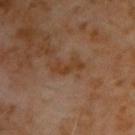This lesion was catalogued during total-body skin photography and was not selected for biopsy.
The lesion is on the right upper arm.
Cropped from a total-body skin-imaging series; the visible field is about 15 mm.
A male patient, aged around 60.